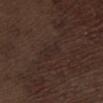Findings:
• image source — total-body-photography crop, ~15 mm field of view
• anatomic site — the left thigh
• diameter — about 2.5 mm
• tile lighting — white-light
• patient — male, aged around 70